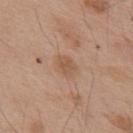| feature | finding |
|---|---|
| image | 15 mm crop, total-body photography |
| patient | male, aged around 55 |
| image-analysis metrics | a lesion area of about 4 mm², a shape eccentricity near 0.7, and two-axis asymmetry of about 0.3; a mean CIELAB color near L≈54 a*≈19 b*≈31 and about 7 CIELAB-L* units darker than the surrounding skin; internal color variation of about 2.5 on a 0–10 scale and radial color variation of about 1; an automated nevus-likeness rating near 0 out of 100 and a detector confidence of about 100 out of 100 that the crop contains a lesion |
| illumination | white-light |
| body site | the back |